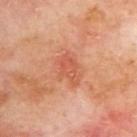Q: Was this lesion biopsied?
A: imaged on a skin check; not biopsied
Q: Lesion size?
A: about 4 mm
Q: How was the tile lit?
A: cross-polarized illumination
Q: What kind of image is this?
A: 15 mm crop, total-body photography
Q: Who is the patient?
A: male, approximately 70 years of age
Q: What did automated image analysis measure?
A: an average lesion color of about L≈56 a*≈29 b*≈35 (CIELAB) and roughly 7 lightness units darker than nearby skin; a color-variation rating of about 2.5/10 and radial color variation of about 1; an automated nevus-likeness rating near 0 out of 100 and a lesion-detection confidence of about 100/100
Q: Where on the body is the lesion?
A: the back